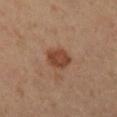{
  "biopsy_status": "not biopsied; imaged during a skin examination",
  "patient": {
    "sex": "male",
    "age_approx": 65
  },
  "lighting": "cross-polarized",
  "site": "leg",
  "image": {
    "source": "total-body photography crop",
    "field_of_view_mm": 15
  },
  "lesion_size": {
    "long_diameter_mm_approx": 3.5
  }
}